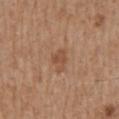No biopsy was performed on this lesion — it was imaged during a full skin examination and was not determined to be concerning. Cropped from a whole-body photographic skin survey; the tile spans about 15 mm. An algorithmic analysis of the crop reported an outline eccentricity of about 0.7 (0 = round, 1 = elongated). And it measured a border-irregularity index near 3/10, a color-variation rating of about 2/10, and radial color variation of about 1. The software also gave a nevus-likeness score of about 0/100. Captured under white-light illumination. The lesion is located on the mid back. Longest diameter approximately 3 mm. A male patient, aged 68–72.